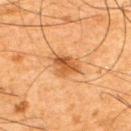Measured at roughly 3.5 mm in maximum diameter.
The lesion is located on the upper back.
Imaged with cross-polarized lighting.
The total-body-photography lesion software estimated an average lesion color of about L≈47 a*≈23 b*≈38 (CIELAB) and a lesion–skin lightness drop of about 10.
A male patient aged 63–67.
A 15 mm close-up tile from a total-body photography series done for melanoma screening.